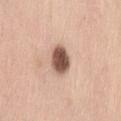follow-up: catalogued during a skin exam; not biopsied | subject: female, aged 53 to 57 | size: ~3.5 mm (longest diameter) | imaging modality: ~15 mm tile from a whole-body skin photo | body site: the left thigh.